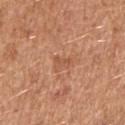Context:
A 15 mm close-up tile from a total-body photography series done for melanoma screening. A female subject, in their 40s. Captured under white-light illumination. The lesion is on the right upper arm. The lesion's longest dimension is about 2.5 mm.